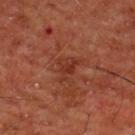Recorded during total-body skin imaging; not selected for excision or biopsy. A region of skin cropped from a whole-body photographic capture, roughly 15 mm wide. From the chest. The tile uses cross-polarized illumination. Measured at roughly 3 mm in maximum diameter. A male subject, aged 58 to 62.